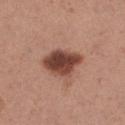Q: Is there a histopathology result?
A: total-body-photography surveillance lesion; no biopsy
Q: What did automated image analysis measure?
A: an area of roughly 14 mm², an eccentricity of roughly 0.7, and a symmetry-axis asymmetry near 0.2; a nevus-likeness score of about 85/100
Q: How was the tile lit?
A: white-light illumination
Q: What kind of image is this?
A: ~15 mm crop, total-body skin-cancer survey
Q: Patient demographics?
A: female, aged approximately 30
Q: What is the anatomic site?
A: the left thigh
Q: What is the lesion's diameter?
A: ≈5 mm